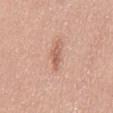workup: catalogued during a skin exam; not biopsied | tile lighting: white-light illumination | site: the mid back | image source: total-body-photography crop, ~15 mm field of view | subject: female, about 45 years old | image-analysis metrics: a footprint of about 2.5 mm² and a shape eccentricity near 0.9; a mean CIELAB color near L≈59 a*≈23 b*≈30 and a normalized border contrast of about 6.5; a border-irregularity rating of about 3/10 and a within-lesion color-variation index near 0/10 | size: ≈2.5 mm.